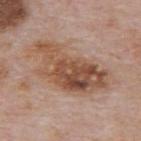| field | value |
|---|---|
| notes | total-body-photography surveillance lesion; no biopsy |
| TBP lesion metrics | a mean CIELAB color near L≈51 a*≈20 b*≈30, a lesion–skin lightness drop of about 12, and a lesion-to-skin contrast of about 9 (normalized; higher = more distinct); border irregularity of about 5 on a 0–10 scale, internal color variation of about 8 on a 0–10 scale, and radial color variation of about 3 |
| lesion size | about 9 mm |
| patient | male, in their mid- to late 70s |
| site | the mid back |
| lighting | white-light illumination |
| acquisition | total-body-photography crop, ~15 mm field of view |